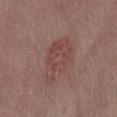The lesion was tiled from a total-body skin photograph and was not biopsied. On the right thigh. Captured under white-light illumination. A roughly 15 mm field-of-view crop from a total-body skin photograph. Measured at roughly 6 mm in maximum diameter. A female patient, in their mid-50s.